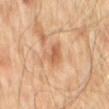Assessment:
Part of a total-body skin-imaging series; this lesion was reviewed on a skin check and was not flagged for biopsy.